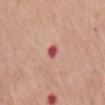Imaged during a routine full-body skin examination; the lesion was not biopsied and no histopathology is available. The recorded lesion diameter is about 2.5 mm. Located on the front of the torso. This is a white-light tile. A female patient, in their mid- to late 60s. A 15 mm crop from a total-body photograph taken for skin-cancer surveillance.